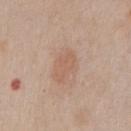site = the chest
lighting = white-light illumination
acquisition = ~15 mm tile from a whole-body skin photo
automated lesion analysis = an average lesion color of about L≈59 a*≈19 b*≈29 (CIELAB), roughly 6 lightness units darker than nearby skin, and a normalized border contrast of about 5; a border-irregularity index near 5/10, a within-lesion color-variation index near 1.5/10, and a peripheral color-asymmetry measure near 0.5; a nevus-likeness score of about 15/100
patient = male, aged 58 to 62